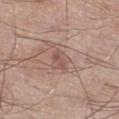No biopsy was performed on this lesion — it was imaged during a full skin examination and was not determined to be concerning.
A close-up tile cropped from a whole-body skin photograph, about 15 mm across.
The lesion is on the leg.
A male patient, aged 68–72.